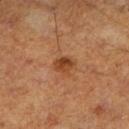Measured at roughly 2.5 mm in maximum diameter. This is a cross-polarized tile. A roughly 15 mm field-of-view crop from a total-body skin photograph. From the right lower leg. The subject is a male aged approximately 60.